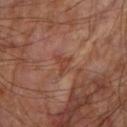Notes:
• size · about 2.5 mm
• imaging modality · total-body-photography crop, ~15 mm field of view
• body site · the left forearm
• patient · male, in their mid- to late 80s or older